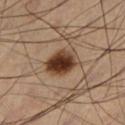<case>
  <biopsy_status>not biopsied; imaged during a skin examination</biopsy_status>
  <automated_metrics>
    <nevus_likeness_0_100>100</nevus_likeness_0_100>
    <lesion_detection_confidence_0_100>100</lesion_detection_confidence_0_100>
  </automated_metrics>
  <lighting>cross-polarized</lighting>
  <lesion_size>
    <long_diameter_mm_approx>4.5</long_diameter_mm_approx>
  </lesion_size>
  <patient>
    <sex>male</sex>
    <age_approx>65</age_approx>
  </patient>
  <site>left lower leg</site>
  <image>
    <source>total-body photography crop</source>
    <field_of_view_mm>15</field_of_view_mm>
  </image>
</case>